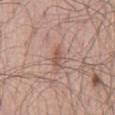Q: Was a biopsy performed?
A: total-body-photography surveillance lesion; no biopsy
Q: Patient demographics?
A: male, in their mid-50s
Q: What is the imaging modality?
A: ~15 mm tile from a whole-body skin photo
Q: What lighting was used for the tile?
A: white-light
Q: Where on the body is the lesion?
A: the chest